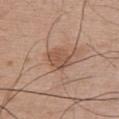follow-up = catalogued during a skin exam; not biopsied
automated lesion analysis = a lesion color around L≈52 a*≈20 b*≈30 in CIELAB and roughly 9 lightness units darker than nearby skin; border irregularity of about 2 on a 0–10 scale, internal color variation of about 3 on a 0–10 scale, and peripheral color asymmetry of about 1; a detector confidence of about 100 out of 100 that the crop contains a lesion
image = ~15 mm crop, total-body skin-cancer survey
subject = male, aged 48 to 52
diameter = about 3.5 mm
tile lighting = white-light illumination
anatomic site = the upper back